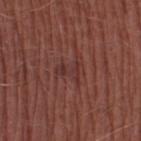{"biopsy_status": "not biopsied; imaged during a skin examination", "lighting": "white-light", "image": {"source": "total-body photography crop", "field_of_view_mm": 15}, "patient": {"sex": "male", "age_approx": 65}, "site": "leg", "lesion_size": {"long_diameter_mm_approx": 3.0}}